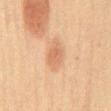biopsy_status: not biopsied; imaged during a skin examination
image:
  source: total-body photography crop
  field_of_view_mm: 15
site: mid back
patient:
  sex: male
  age_approx: 60
lesion_size:
  long_diameter_mm_approx: 4.0
automated_metrics:
  area_mm2_approx: 5.5
  eccentricity: 0.85
  shape_asymmetry: 0.2
  cielab_L: 53
  cielab_a: 19
  cielab_b: 30
  vs_skin_darker_L: 8.0
  vs_skin_contrast_norm: 5.5
  peripheral_color_asymmetry: 0.5